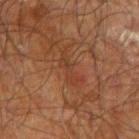This is a cross-polarized tile.
The lesion is on the left forearm.
A close-up tile cropped from a whole-body skin photograph, about 15 mm across.
Measured at roughly 4 mm in maximum diameter.
The total-body-photography lesion software estimated an average lesion color of about L≈32 a*≈20 b*≈27 (CIELAB), roughly 4 lightness units darker than nearby skin, and a lesion-to-skin contrast of about 4.5 (normalized; higher = more distinct). The analysis additionally found a border-irregularity rating of about 7/10. It also reported a detector confidence of about 90 out of 100 that the crop contains a lesion.
A male patient in their 70s.